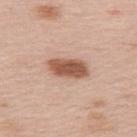Imaged during a routine full-body skin examination; the lesion was not biopsied and no histopathology is available. This image is a 15 mm lesion crop taken from a total-body photograph. This is a white-light tile. Located on the upper back. A female subject, aged around 65. The lesion-visualizer software estimated an outline eccentricity of about 0.9 (0 = round, 1 = elongated). It also reported an average lesion color of about L≈55 a*≈22 b*≈30 (CIELAB) and roughly 16 lightness units darker than nearby skin. The software also gave internal color variation of about 3 on a 0–10 scale.